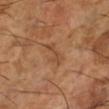Captured during whole-body skin photography for melanoma surveillance; the lesion was not biopsied. From the left lower leg. The subject is a male aged 63–67. This is a cross-polarized tile. A 15 mm crop from a total-body photograph taken for skin-cancer surveillance.